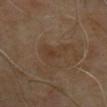| field | value |
|---|---|
| workup | imaged on a skin check; not biopsied |
| tile lighting | cross-polarized |
| imaging modality | ~15 mm tile from a whole-body skin photo |
| site | the left upper arm |
| lesion size | ≈5.5 mm |
| patient | male, aged 63–67 |